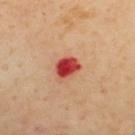No biopsy was performed on this lesion — it was imaged during a full skin examination and was not determined to be concerning.
A 15 mm close-up extracted from a 3D total-body photography capture.
Approximately 3 mm at its widest.
Captured under cross-polarized illumination.
The patient is a male approximately 55 years of age.
From the back.